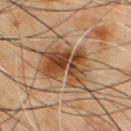{"biopsy_status": "not biopsied; imaged during a skin examination", "site": "front of the torso", "patient": {"sex": "male", "age_approx": 55}, "lighting": "cross-polarized", "lesion_size": {"long_diameter_mm_approx": 6.0}, "image": {"source": "total-body photography crop", "field_of_view_mm": 15}}